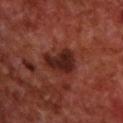Recorded during total-body skin imaging; not selected for excision or biopsy. A 15 mm crop from a total-body photograph taken for skin-cancer surveillance. This is a cross-polarized tile. A male patient about 70 years old. Automated image analysis of the tile measured a lesion color around L≈24 a*≈23 b*≈24 in CIELAB and about 11 CIELAB-L* units darker than the surrounding skin. It also reported a color-variation rating of about 3.5/10. It also reported a nevus-likeness score of about 25/100. From the upper back.